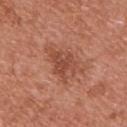follow-up = catalogued during a skin exam; not biopsied
imaging modality = total-body-photography crop, ~15 mm field of view
tile lighting = white-light illumination
lesion size = ≈5.5 mm
location = the upper back
subject = male, about 55 years old
TBP lesion metrics = an area of roughly 11 mm², an eccentricity of roughly 0.8, and two-axis asymmetry of about 0.4